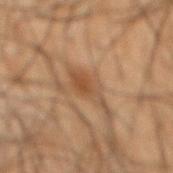– biopsy status · catalogued during a skin exam; not biopsied
– anatomic site · the mid back
– subject · male, aged 48–52
– imaging modality · total-body-photography crop, ~15 mm field of view